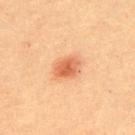Findings:
- follow-up · total-body-photography surveillance lesion; no biopsy
- site · the upper back
- imaging modality · total-body-photography crop, ~15 mm field of view
- TBP lesion metrics · a footprint of about 7.5 mm² and a shape-asymmetry score of about 0.2 (0 = symmetric); a mean CIELAB color near L≈53 a*≈24 b*≈34 and a normalized border contrast of about 7.5; a border-irregularity rating of about 1.5/10 and a peripheral color-asymmetry measure near 1; a lesion-detection confidence of about 100/100
- illumination · cross-polarized
- subject · male, in their 60s
- size · ~3.5 mm (longest diameter)